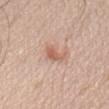– notes: no biopsy performed (imaged during a skin exam)
– lighting: white-light illumination
– acquisition: 15 mm crop, total-body photography
– location: the front of the torso
– subject: male, in their 70s
– diameter: ≈3 mm